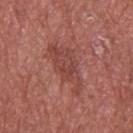Imaged during a routine full-body skin examination; the lesion was not biopsied and no histopathology is available. This image is a 15 mm lesion crop taken from a total-body photograph. A male patient, aged approximately 75. From the upper back.